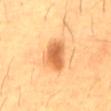Impression:
The lesion was photographed on a routine skin check and not biopsied; there is no pathology result.